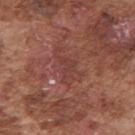Impression: Recorded during total-body skin imaging; not selected for excision or biopsy. Context: On the right upper arm. The recorded lesion diameter is about 4.5 mm. This is a white-light tile. The subject is a male aged 73–77. A 15 mm crop from a total-body photograph taken for skin-cancer surveillance.